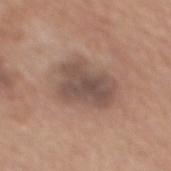biopsy status: no biopsy performed (imaged during a skin exam) | acquisition: 15 mm crop, total-body photography | patient: female, aged 53 to 57 | body site: the mid back.